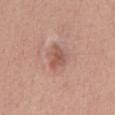The lesion was tiled from a total-body skin photograph and was not biopsied.
The recorded lesion diameter is about 3.5 mm.
From the mid back.
A close-up tile cropped from a whole-body skin photograph, about 15 mm across.
A female patient, about 45 years old.
The tile uses white-light illumination.